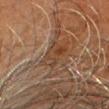biopsy_status: not biopsied; imaged during a skin examination
lighting: cross-polarized
automated_metrics:
  area_mm2_approx: 7.5
  shape_asymmetry: 0.25
patient:
  sex: male
  age_approx: 65
lesion_size:
  long_diameter_mm_approx: 3.5
site: head or neck
image:
  source: total-body photography crop
  field_of_view_mm: 15